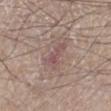<record>
  <biopsy_status>not biopsied; imaged during a skin examination</biopsy_status>
  <automated_metrics>
    <area_mm2_approx>5.0</area_mm2_approx>
    <eccentricity>0.95</eccentricity>
    <shape_asymmetry>0.45</shape_asymmetry>
    <border_irregularity_0_10>5.0</border_irregularity_0_10>
    <color_variation_0_10>1.0</color_variation_0_10>
    <peripheral_color_asymmetry>0.5</peripheral_color_asymmetry>
    <nevus_likeness_0_100>0</nevus_likeness_0_100>
    <lesion_detection_confidence_0_100>80</lesion_detection_confidence_0_100>
  </automated_metrics>
  <lighting>white-light</lighting>
  <image>
    <source>total-body photography crop</source>
    <field_of_view_mm>15</field_of_view_mm>
  </image>
  <lesion_size>
    <long_diameter_mm_approx>4.0</long_diameter_mm_approx>
  </lesion_size>
  <site>left lower leg</site>
  <patient>
    <sex>male</sex>
    <age_approx>75</age_approx>
  </patient>
</record>